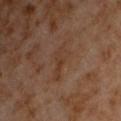follow-up: total-body-photography surveillance lesion; no biopsy | imaging modality: ~15 mm crop, total-body skin-cancer survey | location: the chest | patient: male, aged approximately 60.